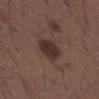Q: Lesion location?
A: the mid back
Q: Who is the patient?
A: male, in their 50s
Q: What kind of image is this?
A: ~15 mm crop, total-body skin-cancer survey
Q: Automated lesion metrics?
A: an automated nevus-likeness rating near 90 out of 100 and a detector confidence of about 100 out of 100 that the crop contains a lesion
Q: Lesion size?
A: about 4.5 mm
Q: Illumination type?
A: white-light